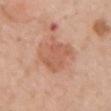No biopsy was performed on this lesion — it was imaged during a full skin examination and was not determined to be concerning. A female patient, in their mid-60s. The lesion is on the left upper arm. Cropped from a total-body skin-imaging series; the visible field is about 15 mm.